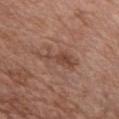Q: Is there a histopathology result?
A: catalogued during a skin exam; not biopsied
Q: Who is the patient?
A: male, in their mid- to late 60s
Q: Lesion location?
A: the chest
Q: What is the imaging modality?
A: total-body-photography crop, ~15 mm field of view